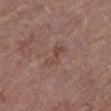Captured during whole-body skin photography for melanoma surveillance; the lesion was not biopsied. Automated tile analysis of the lesion measured a lesion area of about 4 mm², an outline eccentricity of about 0.85 (0 = round, 1 = elongated), and a shape-asymmetry score of about 0.45 (0 = symmetric). The analysis additionally found an automated nevus-likeness rating near 0 out of 100 and a detector confidence of about 100 out of 100 that the crop contains a lesion. A roughly 15 mm field-of-view crop from a total-body skin photograph. Captured under white-light illumination. The recorded lesion diameter is about 3 mm. A female subject aged around 80. The lesion is on the left lower leg.